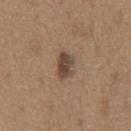follow-up: catalogued during a skin exam; not biopsied
patient: male, in their mid- to late 60s
acquisition: total-body-photography crop, ~15 mm field of view
body site: the chest